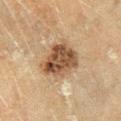The lesion was tiled from a total-body skin photograph and was not biopsied. A 15 mm close-up extracted from a 3D total-body photography capture. The lesion is located on the right lower leg. About 5 mm across. A male subject, aged 58–62. This is a cross-polarized tile.